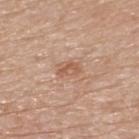Imaged during a routine full-body skin examination; the lesion was not biopsied and no histopathology is available. The lesion is located on the upper back. A male subject roughly 75 years of age. A close-up tile cropped from a whole-body skin photograph, about 15 mm across. Imaged with white-light lighting.